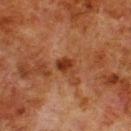{"biopsy_status": "not biopsied; imaged during a skin examination", "image": {"source": "total-body photography crop", "field_of_view_mm": 15}, "patient": {"sex": "male", "age_approx": 80}, "site": "upper back", "lighting": "cross-polarized"}